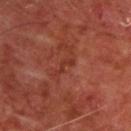A male subject about 65 years old.
Located on the back.
Cropped from a whole-body photographic skin survey; the tile spans about 15 mm.